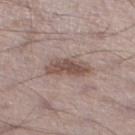Located on the left lower leg.
Automated tile analysis of the lesion measured a mean CIELAB color near L≈50 a*≈16 b*≈22, a lesion–skin lightness drop of about 11, and a normalized border contrast of about 8.
A region of skin cropped from a whole-body photographic capture, roughly 15 mm wide.
Imaged with white-light lighting.
A male patient, aged 68 to 72.